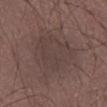biopsy status: total-body-photography surveillance lesion; no biopsy
body site: the abdomen
TBP lesion metrics: a lesion–skin lightness drop of about 5 and a lesion-to-skin contrast of about 4.5 (normalized; higher = more distinct); a border-irregularity rating of about 4.5/10, internal color variation of about 2 on a 0–10 scale, and peripheral color asymmetry of about 1; an automated nevus-likeness rating near 0 out of 100 and a detector confidence of about 100 out of 100 that the crop contains a lesion
imaging modality: ~15 mm crop, total-body skin-cancer survey
subject: male, aged around 60
tile lighting: white-light
lesion size: about 7.5 mm